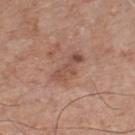No biopsy was performed on this lesion — it was imaged during a full skin examination and was not determined to be concerning.
The patient is a male aged around 75.
A lesion tile, about 15 mm wide, cut from a 3D total-body photograph.
The lesion is located on the chest.
Measured at roughly 4 mm in maximum diameter.